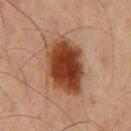Q: Was a biopsy performed?
A: total-body-photography surveillance lesion; no biopsy
Q: What kind of image is this?
A: ~15 mm tile from a whole-body skin photo
Q: Where on the body is the lesion?
A: the chest
Q: How large is the lesion?
A: ~7 mm (longest diameter)
Q: What are the patient's age and sex?
A: male, in their 60s
Q: Illumination type?
A: cross-polarized illumination
Q: What did automated image analysis measure?
A: a mean CIELAB color near L≈38 a*≈23 b*≈31 and a normalized lesion–skin contrast near 13.5